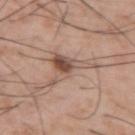Assessment:
This lesion was catalogued during total-body skin photography and was not selected for biopsy.
Clinical summary:
A male subject, aged 53–57. Approximately 7 mm at its widest. Cropped from a whole-body photographic skin survey; the tile spans about 15 mm. This is a white-light tile. The lesion is located on the left upper arm.